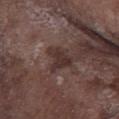Context:
A 15 mm close-up tile from a total-body photography series done for melanoma screening. The lesion is located on the leg. Longest diameter approximately 3.5 mm. An algorithmic analysis of the crop reported a footprint of about 6.5 mm² and a shape-asymmetry score of about 0.55 (0 = symmetric). The software also gave border irregularity of about 6 on a 0–10 scale and radial color variation of about 0.5. It also reported a classifier nevus-likeness of about 0/100 and a detector confidence of about 70 out of 100 that the crop contains a lesion. This is a white-light tile. A male subject, aged approximately 75.